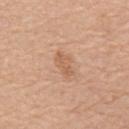Clinical impression:
The lesion was photographed on a routine skin check and not biopsied; there is no pathology result.
Context:
Imaged with white-light lighting. The patient is a male aged around 65. On the abdomen. Cropped from a total-body skin-imaging series; the visible field is about 15 mm.